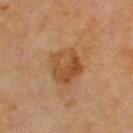workup = imaged on a skin check; not biopsied
imaging modality = ~15 mm tile from a whole-body skin photo
anatomic site = the upper back